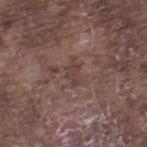This lesion was catalogued during total-body skin photography and was not selected for biopsy.